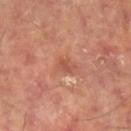Assessment:
The lesion was photographed on a routine skin check and not biopsied; there is no pathology result.
Context:
From the right lower leg. A close-up tile cropped from a whole-body skin photograph, about 15 mm across. A male patient aged 63–67.